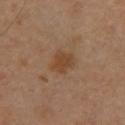Context:
A lesion tile, about 15 mm wide, cut from a 3D total-body photograph. A female subject aged 33–37. Located on the back. An algorithmic analysis of the crop reported a footprint of about 7 mm², an outline eccentricity of about 0.6 (0 = round, 1 = elongated), and two-axis asymmetry of about 0.25. The analysis additionally found a border-irregularity rating of about 2.5/10 and a color-variation rating of about 2/10.